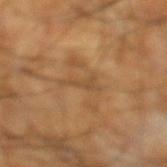Clinical summary: Measured at roughly 4 mm in maximum diameter. A male patient, aged around 60. Cropped from a total-body skin-imaging series; the visible field is about 15 mm. On the left lower leg. This is a cross-polarized tile. The lesion-visualizer software estimated an eccentricity of roughly 0.95 and two-axis asymmetry of about 0.7.